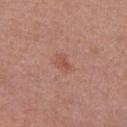{
  "lesion_size": {
    "long_diameter_mm_approx": 2.5
  },
  "image": {
    "source": "total-body photography crop",
    "field_of_view_mm": 15
  },
  "patient": {
    "sex": "female",
    "age_approx": 50
  },
  "automated_metrics": {
    "cielab_L": 53,
    "cielab_a": 24,
    "cielab_b": 29,
    "vs_skin_contrast_norm": 5.0,
    "lesion_detection_confidence_0_100": 100
  },
  "lighting": "white-light",
  "site": "chest"
}